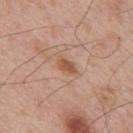Q: Was a biopsy performed?
A: total-body-photography surveillance lesion; no biopsy
Q: How was the tile lit?
A: white-light illumination
Q: What are the patient's age and sex?
A: male, in their mid-60s
Q: Where on the body is the lesion?
A: the mid back
Q: Automated lesion metrics?
A: a border-irregularity index near 2.5/10 and radial color variation of about 0.5
Q: What is the imaging modality?
A: total-body-photography crop, ~15 mm field of view